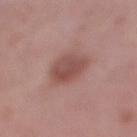This lesion was catalogued during total-body skin photography and was not selected for biopsy. A 15 mm close-up extracted from a 3D total-body photography capture. A female subject, about 50 years old. From the right lower leg.